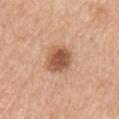Assessment: The lesion was photographed on a routine skin check and not biopsied; there is no pathology result. Acquisition and patient details: Measured at roughly 3.5 mm in maximum diameter. The patient is a female in their mid- to late 60s. A 15 mm close-up tile from a total-body photography series done for melanoma screening. The lesion is on the right upper arm.